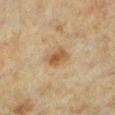Assessment: Part of a total-body skin-imaging series; this lesion was reviewed on a skin check and was not flagged for biopsy. Clinical summary: A female subject, roughly 55 years of age. Automated tile analysis of the lesion measured an average lesion color of about L≈46 a*≈16 b*≈32 (CIELAB), a lesion–skin lightness drop of about 9, and a normalized border contrast of about 7.5. And it measured border irregularity of about 3 on a 0–10 scale and a peripheral color-asymmetry measure near 1. The analysis additionally found a classifier nevus-likeness of about 75/100 and lesion-presence confidence of about 100/100. A lesion tile, about 15 mm wide, cut from a 3D total-body photograph. From the left lower leg.